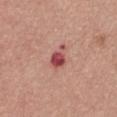- workup · no biopsy performed (imaged during a skin exam)
- image-analysis metrics · a within-lesion color-variation index near 4/10 and radial color variation of about 1.5
- illumination · white-light illumination
- lesion size · about 3 mm
- patient · female, aged around 35
- body site · the chest
- acquisition · ~15 mm crop, total-body skin-cancer survey